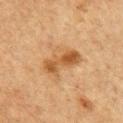Assessment:
Captured during whole-body skin photography for melanoma surveillance; the lesion was not biopsied.
Background:
A male patient in their mid-70s. Longest diameter approximately 5 mm. A lesion tile, about 15 mm wide, cut from a 3D total-body photograph. Located on the front of the torso. The lesion-visualizer software estimated a footprint of about 8.5 mm² and a symmetry-axis asymmetry near 0.25. The analysis additionally found a lesion–skin lightness drop of about 10. The analysis additionally found border irregularity of about 3.5 on a 0–10 scale, a within-lesion color-variation index near 4.5/10, and peripheral color asymmetry of about 1.5.